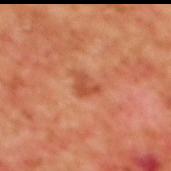No biopsy was performed on this lesion — it was imaged during a full skin examination and was not determined to be concerning.
The recorded lesion diameter is about 3 mm.
The subject is a female in their 40s.
The lesion is on the upper back.
A 15 mm close-up tile from a total-body photography series done for melanoma screening.
Imaged with cross-polarized lighting.